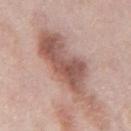{"biopsy_status": "not biopsied; imaged during a skin examination", "lesion_size": {"long_diameter_mm_approx": 10.0}, "image": {"source": "total-body photography crop", "field_of_view_mm": 15}, "automated_metrics": {"area_mm2_approx": 29.0, "shape_asymmetry": 0.35, "vs_skin_darker_L": 13.0, "vs_skin_contrast_norm": 8.5, "color_variation_0_10": 6.0, "peripheral_color_asymmetry": 2.0}, "patient": {"sex": "male", "age_approx": 75}, "site": "mid back", "lighting": "white-light"}